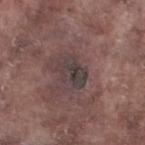Q: Is there a histopathology result?
A: catalogued during a skin exam; not biopsied
Q: Where on the body is the lesion?
A: the left lower leg
Q: Patient demographics?
A: male, aged approximately 75
Q: What lighting was used for the tile?
A: white-light
Q: What kind of image is this?
A: total-body-photography crop, ~15 mm field of view
Q: What did automated image analysis measure?
A: a lesion area of about 7 mm², an outline eccentricity of about 0.7 (0 = round, 1 = elongated), and a shape-asymmetry score of about 0.3 (0 = symmetric); border irregularity of about 3.5 on a 0–10 scale, internal color variation of about 5 on a 0–10 scale, and a peripheral color-asymmetry measure near 1.5